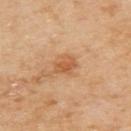notes=imaged on a skin check; not biopsied
imaging modality=~15 mm crop, total-body skin-cancer survey
body site=the upper back
patient=female, in their 60s
diameter=≈3 mm